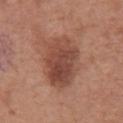The lesion was photographed on a routine skin check and not biopsied; there is no pathology result.
Imaged with white-light lighting.
Approximately 6.5 mm at its widest.
The patient is a male approximately 65 years of age.
A close-up tile cropped from a whole-body skin photograph, about 15 mm across.
Located on the front of the torso.